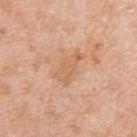Recorded during total-body skin imaging; not selected for excision or biopsy. A 15 mm close-up tile from a total-body photography series done for melanoma screening. Captured under white-light illumination. The lesion is on the right upper arm. Automated image analysis of the tile measured a footprint of about 6 mm². The analysis additionally found a border-irregularity index near 4.5/10, internal color variation of about 1.5 on a 0–10 scale, and peripheral color asymmetry of about 0.5. A male subject, approximately 65 years of age. Approximately 4 mm at its widest.